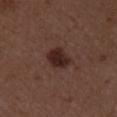{
  "biopsy_status": "not biopsied; imaged during a skin examination",
  "patient": {
    "sex": "female",
    "age_approx": 50
  },
  "image": {
    "source": "total-body photography crop",
    "field_of_view_mm": 15
  },
  "automated_metrics": {
    "cielab_L": 25,
    "cielab_a": 18,
    "cielab_b": 20,
    "vs_skin_darker_L": 10.0,
    "vs_skin_contrast_norm": 10.5,
    "border_irregularity_0_10": 2.0,
    "color_variation_0_10": 4.0,
    "nevus_likeness_0_100": 90,
    "lesion_detection_confidence_0_100": 100
  },
  "site": "right upper arm"
}